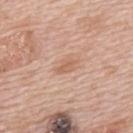A male patient aged 68 to 72. Approximately 2.5 mm at its widest. The lesion-visualizer software estimated border irregularity of about 3 on a 0–10 scale and internal color variation of about 1.5 on a 0–10 scale. The software also gave an automated nevus-likeness rating near 5 out of 100 and a detector confidence of about 100 out of 100 that the crop contains a lesion. A roughly 15 mm field-of-view crop from a total-body skin photograph. From the upper back. The tile uses white-light illumination.